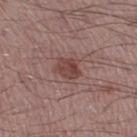notes = no biopsy performed (imaged during a skin exam); anatomic site = the right thigh; image source = 15 mm crop, total-body photography; diameter = ≈3 mm; patient = male, roughly 50 years of age.